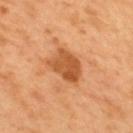Captured during whole-body skin photography for melanoma surveillance; the lesion was not biopsied.
Cropped from a whole-body photographic skin survey; the tile spans about 15 mm.
From the upper back.
The patient is a male approximately 50 years of age.